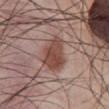notes: imaged on a skin check; not biopsied | patient: male, approximately 55 years of age | lighting: white-light | acquisition: 15 mm crop, total-body photography | site: the abdomen | diameter: ≈4 mm.